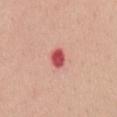notes = total-body-photography surveillance lesion; no biopsy
site = the mid back
patient = female, aged 38–42
automated metrics = border irregularity of about 2 on a 0–10 scale and a color-variation rating of about 2.5/10; a classifier nevus-likeness of about 0/100 and a detector confidence of about 100 out of 100 that the crop contains a lesion
lesion diameter = about 2.5 mm
tile lighting = white-light illumination
image source = total-body-photography crop, ~15 mm field of view